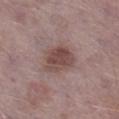Captured during whole-body skin photography for melanoma surveillance; the lesion was not biopsied. A male subject, in their mid-70s. From the right lower leg. This image is a 15 mm lesion crop taken from a total-body photograph.